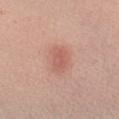Imaged during a routine full-body skin examination; the lesion was not biopsied and no histopathology is available. An algorithmic analysis of the crop reported roughly 8 lightness units darker than nearby skin. The software also gave a border-irregularity index near 2/10 and a within-lesion color-variation index near 2.5/10. A region of skin cropped from a whole-body photographic capture, roughly 15 mm wide. Measured at roughly 3.5 mm in maximum diameter. From the left forearm. A male subject approximately 40 years of age.